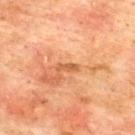| feature | finding |
|---|---|
| biopsy status | imaged on a skin check; not biopsied |
| patient | male, aged 73–77 |
| lesion size | ~2.5 mm (longest diameter) |
| location | the upper back |
| lighting | cross-polarized |
| image source | 15 mm crop, total-body photography |